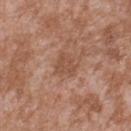{
  "lesion_size": {
    "long_diameter_mm_approx": 2.5
  },
  "site": "back",
  "automated_metrics": {
    "area_mm2_approx": 5.0,
    "eccentricity": 0.5,
    "border_irregularity_0_10": 4.0,
    "color_variation_0_10": 2.0,
    "nevus_likeness_0_100": 0,
    "lesion_detection_confidence_0_100": 100
  },
  "patient": {
    "sex": "male",
    "age_approx": 45
  },
  "image": {
    "source": "total-body photography crop",
    "field_of_view_mm": 15
  }
}